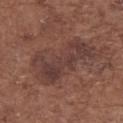notes = no biopsy performed (imaged during a skin exam)
TBP lesion metrics = a border-irregularity rating of about 8.5/10, internal color variation of about 3.5 on a 0–10 scale, and a peripheral color-asymmetry measure near 1; an automated nevus-likeness rating near 0 out of 100 and lesion-presence confidence of about 100/100
patient = female, approximately 75 years of age
illumination = white-light illumination
image source = total-body-photography crop, ~15 mm field of view
lesion diameter = ≈7.5 mm
location = the upper back